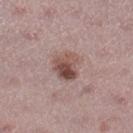{
  "biopsy_status": "not biopsied; imaged during a skin examination",
  "lighting": "white-light",
  "automated_metrics": {
    "shape_asymmetry": 0.25,
    "cielab_L": 50,
    "cielab_a": 19,
    "cielab_b": 22,
    "vs_skin_darker_L": 12.0,
    "vs_skin_contrast_norm": 8.5,
    "border_irregularity_0_10": 2.5,
    "color_variation_0_10": 8.0,
    "nevus_likeness_0_100": 75
  },
  "site": "right lower leg",
  "lesion_size": {
    "long_diameter_mm_approx": 3.5
  },
  "patient": {
    "sex": "female",
    "age_approx": 45
  },
  "image": {
    "source": "total-body photography crop",
    "field_of_view_mm": 15
  }
}